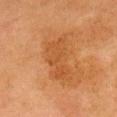follow-up = no biopsy performed (imaged during a skin exam)
image-analysis metrics = a within-lesion color-variation index near 2.5/10 and peripheral color asymmetry of about 1
location = the head or neck
lesion diameter = ~6 mm (longest diameter)
patient = female, aged around 50
imaging modality = ~15 mm tile from a whole-body skin photo
tile lighting = cross-polarized